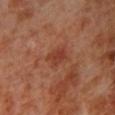Clinical impression:
Recorded during total-body skin imaging; not selected for excision or biopsy.
Acquisition and patient details:
From the left lower leg. This image is a 15 mm lesion crop taken from a total-body photograph. A male subject, in their 70s. Imaged with cross-polarized lighting. Automated tile analysis of the lesion measured a lesion area of about 5 mm² and a symmetry-axis asymmetry near 0.25. And it measured a lesion color around L≈39 a*≈27 b*≈30 in CIELAB, about 7 CIELAB-L* units darker than the surrounding skin, and a lesion-to-skin contrast of about 6.5 (normalized; higher = more distinct). And it measured a color-variation rating of about 2.5/10.